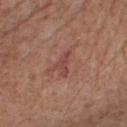patient: male, in their mid- to late 50s | image source: ~15 mm crop, total-body skin-cancer survey | anatomic site: the chest.